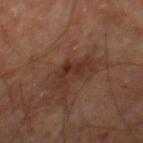<record>
<biopsy_status>not biopsied; imaged during a skin examination</biopsy_status>
<site>right leg</site>
<patient>
  <sex>male</sex>
  <age_approx>60</age_approx>
</patient>
<lighting>cross-polarized</lighting>
<lesion_size>
  <long_diameter_mm_approx>4.0</long_diameter_mm_approx>
</lesion_size>
<automated_metrics>
  <area_mm2_approx>5.5</area_mm2_approx>
  <eccentricity>0.85</eccentricity>
  <shape_asymmetry>0.6</shape_asymmetry>
  <color_variation_0_10>2.0</color_variation_0_10>
  <peripheral_color_asymmetry>0.5</peripheral_color_asymmetry>
</automated_metrics>
<image>
  <source>total-body photography crop</source>
  <field_of_view_mm>15</field_of_view_mm>
</image>
</record>